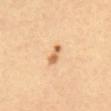<lesion>
<biopsy_status>not biopsied; imaged during a skin examination</biopsy_status>
<lesion_size>
  <long_diameter_mm_approx>3.0</long_diameter_mm_approx>
</lesion_size>
<lighting>cross-polarized</lighting>
<patient>
  <sex>female</sex>
  <age_approx>50</age_approx>
</patient>
<automated_metrics>
  <vs_skin_darker_L>13.0</vs_skin_darker_L>
  <border_irregularity_0_10>3.0</border_irregularity_0_10>
  <peripheral_color_asymmetry>0.0</peripheral_color_asymmetry>
  <nevus_likeness_0_100>80</nevus_likeness_0_100>
  <lesion_detection_confidence_0_100>100</lesion_detection_confidence_0_100>
</automated_metrics>
<image>
  <source>total-body photography crop</source>
  <field_of_view_mm>15</field_of_view_mm>
</image>
</lesion>